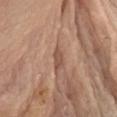Impression: Recorded during total-body skin imaging; not selected for excision or biopsy. Context: A male subject roughly 80 years of age. Imaged with white-light lighting. Approximately 2.5 mm at its widest. Cropped from a total-body skin-imaging series; the visible field is about 15 mm. On the chest.